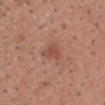This lesion was catalogued during total-body skin photography and was not selected for biopsy. On the chest. A male patient, roughly 55 years of age. Captured under white-light illumination. A 15 mm close-up tile from a total-body photography series done for melanoma screening.